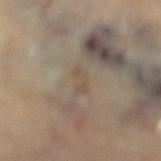Q: Was a biopsy performed?
A: imaged on a skin check; not biopsied
Q: Patient demographics?
A: female, aged around 60
Q: What is the anatomic site?
A: the left lower leg
Q: What kind of image is this?
A: ~15 mm tile from a whole-body skin photo
Q: Illumination type?
A: cross-polarized illumination